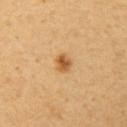This lesion was catalogued during total-body skin photography and was not selected for biopsy. This is a cross-polarized tile. A female patient roughly 35 years of age. On the arm. A 15 mm crop from a total-body photograph taken for skin-cancer surveillance.